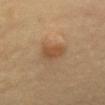Assessment:
Recorded during total-body skin imaging; not selected for excision or biopsy.
Background:
This image is a 15 mm lesion crop taken from a total-body photograph. Captured under cross-polarized illumination. About 3.5 mm across. Located on the front of the torso. A female subject aged 43–47.